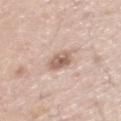Imaged during a routine full-body skin examination; the lesion was not biopsied and no histopathology is available. A male patient in their 40s. Captured under white-light illumination. On the arm. Cropped from a whole-body photographic skin survey; the tile spans about 15 mm. About 2.5 mm across.